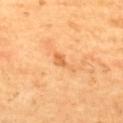Recorded during total-body skin imaging; not selected for excision or biopsy. Cropped from a total-body skin-imaging series; the visible field is about 15 mm. Longest diameter approximately 3 mm. A male patient, in their 60s. Imaged with cross-polarized lighting. From the upper back. Automated image analysis of the tile measured a footprint of about 3 mm² and two-axis asymmetry of about 0.35. The software also gave a border-irregularity rating of about 4/10, a color-variation rating of about 1.5/10, and a peripheral color-asymmetry measure near 0.5.